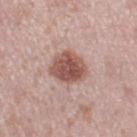Q: Was a biopsy performed?
A: catalogued during a skin exam; not biopsied
Q: What kind of image is this?
A: total-body-photography crop, ~15 mm field of view
Q: Lesion location?
A: the left thigh
Q: How large is the lesion?
A: ~4 mm (longest diameter)
Q: Automated lesion metrics?
A: an area of roughly 11 mm², a shape eccentricity near 0.6, and a shape-asymmetry score of about 0.15 (0 = symmetric); a border-irregularity index near 1.5/10, a color-variation rating of about 4/10, and a peripheral color-asymmetry measure near 1.5; a classifier nevus-likeness of about 90/100
Q: How was the tile lit?
A: white-light
Q: What are the patient's age and sex?
A: female, in their 40s